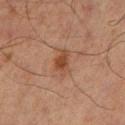The lesion was photographed on a routine skin check and not biopsied; there is no pathology result. Located on the leg. The patient is a male aged 63–67. Imaged with cross-polarized lighting. The total-body-photography lesion software estimated an area of roughly 5 mm², an outline eccentricity of about 0.8 (0 = round, 1 = elongated), and two-axis asymmetry of about 0.3. And it measured an automated nevus-likeness rating near 75 out of 100 and a detector confidence of about 100 out of 100 that the crop contains a lesion. A 15 mm crop from a total-body photograph taken for skin-cancer surveillance.